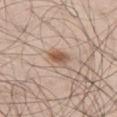Recorded during total-body skin imaging; not selected for excision or biopsy. The lesion is on the left thigh. A 15 mm close-up tile from a total-body photography series done for melanoma screening. A male subject in their mid-40s.